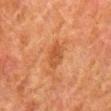Recorded during total-body skin imaging; not selected for excision or biopsy. A male subject, aged 78 to 82. Cropped from a total-body skin-imaging series; the visible field is about 15 mm. The lesion is located on the right lower leg. Automated tile analysis of the lesion measured an average lesion color of about L≈41 a*≈24 b*≈34 (CIELAB), a lesion–skin lightness drop of about 7, and a lesion-to-skin contrast of about 6 (normalized; higher = more distinct). The software also gave a border-irregularity index near 2/10 and a within-lesion color-variation index near 2.5/10.